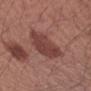Imaged during a routine full-body skin examination; the lesion was not biopsied and no histopathology is available. Longest diameter approximately 4.5 mm. On the left forearm. A region of skin cropped from a whole-body photographic capture, roughly 15 mm wide. A female subject approximately 50 years of age. Captured under white-light illumination.